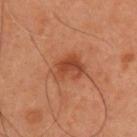follow-up = no biopsy performed (imaged during a skin exam)
tile lighting = cross-polarized illumination
image source = ~15 mm tile from a whole-body skin photo
automated lesion analysis = a mean CIELAB color near L≈45 a*≈27 b*≈34 and roughly 9 lightness units darker than nearby skin; a color-variation rating of about 5.5/10 and a peripheral color-asymmetry measure near 2; an automated nevus-likeness rating near 85 out of 100
patient = male, approximately 55 years of age
diameter = about 3.5 mm
anatomic site = the upper back